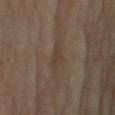workup: no biopsy performed (imaged during a skin exam)
automated lesion analysis: a lesion color around L≈37 a*≈12 b*≈22 in CIELAB, about 5 CIELAB-L* units darker than the surrounding skin, and a lesion-to-skin contrast of about 5 (normalized; higher = more distinct); a border-irregularity index near 3.5/10, a color-variation rating of about 0.5/10, and peripheral color asymmetry of about 0
subject: male, aged around 70
anatomic site: the right upper arm
image: ~15 mm crop, total-body skin-cancer survey